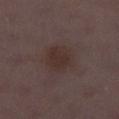Recorded during total-body skin imaging; not selected for excision or biopsy. The total-body-photography lesion software estimated a shape eccentricity near 0.4 and a shape-asymmetry score of about 0.15 (0 = symmetric). The software also gave a border-irregularity index near 1.5/10 and a peripheral color-asymmetry measure near 0.5. It also reported a lesion-detection confidence of about 100/100. A lesion tile, about 15 mm wide, cut from a 3D total-body photograph. The lesion is located on the left lower leg. Imaged with white-light lighting. The recorded lesion diameter is about 4 mm. A female subject, aged 28 to 32.